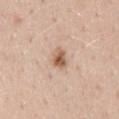Captured during whole-body skin photography for melanoma surveillance; the lesion was not biopsied. A 15 mm close-up extracted from a 3D total-body photography capture. Located on the mid back. The subject is a female aged 38 to 42.